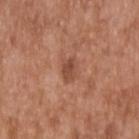Recorded during total-body skin imaging; not selected for excision or biopsy. Located on the upper back. A 15 mm close-up extracted from a 3D total-body photography capture. A male patient, about 65 years old. Approximately 2.5 mm at its widest.